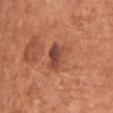notes — total-body-photography surveillance lesion; no biopsy
patient — male, approximately 55 years of age
anatomic site — the chest
lesion diameter — ~3 mm (longest diameter)
image-analysis metrics — an outline eccentricity of about 0.5 (0 = round, 1 = elongated) and a symmetry-axis asymmetry near 0.25; about 11 CIELAB-L* units darker than the surrounding skin and a normalized lesion–skin contrast near 8; a border-irregularity rating of about 3/10, internal color variation of about 6.5 on a 0–10 scale, and a peripheral color-asymmetry measure near 2; a classifier nevus-likeness of about 15/100 and a lesion-detection confidence of about 100/100
acquisition — 15 mm crop, total-body photography